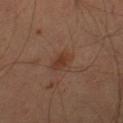<lesion>
  <biopsy_status>not biopsied; imaged during a skin examination</biopsy_status>
  <automated_metrics>
    <area_mm2_approx>4.5</area_mm2_approx>
    <eccentricity>0.5</eccentricity>
    <shape_asymmetry>0.15</shape_asymmetry>
    <cielab_L>36</cielab_L>
    <cielab_a>19</cielab_a>
    <cielab_b>27</cielab_b>
    <vs_skin_darker_L>7.0</vs_skin_darker_L>
    <vs_skin_contrast_norm>6.5</vs_skin_contrast_norm>
  </automated_metrics>
  <site>right thigh</site>
  <patient>
    <sex>male</sex>
    <age_approx>55</age_approx>
  </patient>
  <image>
    <source>total-body photography crop</source>
    <field_of_view_mm>15</field_of_view_mm>
  </image>
  <lighting>cross-polarized</lighting>
</lesion>